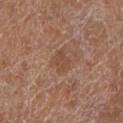| field | value |
|---|---|
| follow-up | imaged on a skin check; not biopsied |
| body site | the left lower leg |
| subject | female, approximately 80 years of age |
| automated lesion analysis | an area of roughly 4.5 mm²; border irregularity of about 2.5 on a 0–10 scale and internal color variation of about 1.5 on a 0–10 scale; a nevus-likeness score of about 0/100 and a detector confidence of about 100 out of 100 that the crop contains a lesion |
| tile lighting | white-light illumination |
| image source | total-body-photography crop, ~15 mm field of view |
| size | ~2.5 mm (longest diameter) |